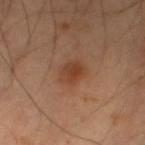Q: Is there a histopathology result?
A: catalogued during a skin exam; not biopsied
Q: What are the patient's age and sex?
A: male, aged around 40
Q: What did automated image analysis measure?
A: a mean CIELAB color near L≈41 a*≈23 b*≈32, roughly 8 lightness units darker than nearby skin, and a normalized lesion–skin contrast near 7; a border-irregularity index near 2/10, a within-lesion color-variation index near 3.5/10, and a peripheral color-asymmetry measure near 1; an automated nevus-likeness rating near 80 out of 100 and lesion-presence confidence of about 100/100
Q: Illumination type?
A: cross-polarized illumination
Q: How was this image acquired?
A: total-body-photography crop, ~15 mm field of view
Q: Lesion size?
A: about 3 mm
Q: Where on the body is the lesion?
A: the left thigh